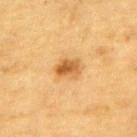Captured during whole-body skin photography for melanoma surveillance; the lesion was not biopsied. A lesion tile, about 15 mm wide, cut from a 3D total-body photograph. The lesion-visualizer software estimated border irregularity of about 2.5 on a 0–10 scale, internal color variation of about 5.5 on a 0–10 scale, and a peripheral color-asymmetry measure near 2. A male patient in their mid-80s or older. The lesion is located on the upper back. The tile uses cross-polarized illumination. About 3 mm across.